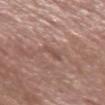This lesion was catalogued during total-body skin photography and was not selected for biopsy. A lesion tile, about 15 mm wide, cut from a 3D total-body photograph. This is a white-light tile. An algorithmic analysis of the crop reported a color-variation rating of about 1.5/10 and a peripheral color-asymmetry measure near 0.5. It also reported a nevus-likeness score of about 0/100 and a lesion-detection confidence of about 100/100. From the left forearm. The lesion's longest dimension is about 2.5 mm. A male subject in their mid- to late 50s.